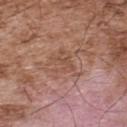notes — imaged on a skin check; not biopsied | patient — male, approximately 55 years of age | location — the upper back | imaging modality — ~15 mm tile from a whole-body skin photo.